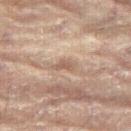| feature | finding |
|---|---|
| notes | imaged on a skin check; not biopsied |
| image | total-body-photography crop, ~15 mm field of view |
| subject | female, roughly 80 years of age |
| location | the left leg |
| size | about 2.5 mm |